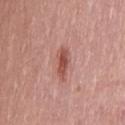{
  "biopsy_status": "not biopsied; imaged during a skin examination",
  "site": "left thigh",
  "lighting": "white-light",
  "image": {
    "source": "total-body photography crop",
    "field_of_view_mm": 15
  },
  "patient": {
    "sex": "female",
    "age_approx": 40
  }
}